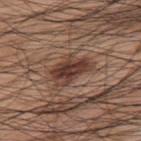Assessment: Captured during whole-body skin photography for melanoma surveillance; the lesion was not biopsied. Clinical summary: A 15 mm close-up tile from a total-body photography series done for melanoma screening. Captured under white-light illumination. The total-body-photography lesion software estimated a footprint of about 10 mm² and an eccentricity of roughly 0.85. The analysis additionally found a lesion color around L≈38 a*≈20 b*≈24 in CIELAB, a lesion–skin lightness drop of about 12, and a normalized lesion–skin contrast near 10. The analysis additionally found a border-irregularity rating of about 4/10 and a peripheral color-asymmetry measure near 1.5. On the back. The recorded lesion diameter is about 5 mm. A male patient aged around 70.